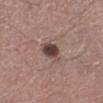  biopsy_status: not biopsied; imaged during a skin examination
  automated_metrics:
    cielab_L: 43
    cielab_a: 17
    cielab_b: 19
    lesion_detection_confidence_0_100: 100
  lesion_size:
    long_diameter_mm_approx: 3.0
  patient:
    sex: male
    age_approx: 45
  site: left lower leg
  image:
    source: total-body photography crop
    field_of_view_mm: 15